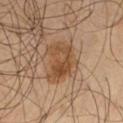<record>
  <image>
    <source>total-body photography crop</source>
    <field_of_view_mm>15</field_of_view_mm>
  </image>
  <patient>
    <sex>male</sex>
    <age_approx>55</age_approx>
  </patient>
  <site>leg</site>
</record>